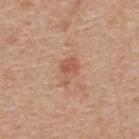Clinical impression:
No biopsy was performed on this lesion — it was imaged during a full skin examination and was not determined to be concerning.
Acquisition and patient details:
The lesion is located on the upper back. A male subject approximately 55 years of age. Imaged with white-light lighting. A lesion tile, about 15 mm wide, cut from a 3D total-body photograph. The lesion's longest dimension is about 3.5 mm.